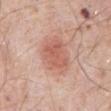Image and clinical context:
Automated image analysis of the tile measured a footprint of about 15 mm², a shape eccentricity near 0.7, and a shape-asymmetry score of about 0.2 (0 = symmetric). It also reported an average lesion color of about L≈60 a*≈25 b*≈29 (CIELAB), a lesion–skin lightness drop of about 9, and a normalized lesion–skin contrast near 6.5. And it measured a classifier nevus-likeness of about 95/100 and lesion-presence confidence of about 100/100. The subject is a male approximately 80 years of age. Cropped from a whole-body photographic skin survey; the tile spans about 15 mm. Longest diameter approximately 5 mm. From the front of the torso.